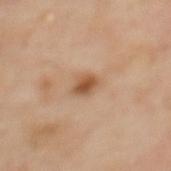biopsy status = catalogued during a skin exam; not biopsied | body site = the upper back | size = about 2.5 mm | image source = 15 mm crop, total-body photography | patient = female, aged 58–62 | lighting = cross-polarized.